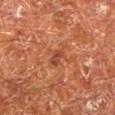<lesion>
<biopsy_status>not biopsied; imaged during a skin examination</biopsy_status>
<lesion_size>
  <long_diameter_mm_approx>2.5</long_diameter_mm_approx>
</lesion_size>
<patient>
  <sex>male</sex>
  <age_approx>65</age_approx>
</patient>
<site>right lower leg</site>
<image>
  <source>total-body photography crop</source>
  <field_of_view_mm>15</field_of_view_mm>
</image>
</lesion>